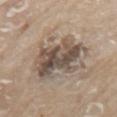notes — catalogued during a skin exam; not biopsied
illumination — white-light
patient — male, aged around 80
anatomic site — the left upper arm
acquisition — total-body-photography crop, ~15 mm field of view